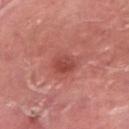Impression:
The lesion was tiled from a total-body skin photograph and was not biopsied.
Background:
About 2.5 mm across. A 15 mm close-up tile from a total-body photography series done for melanoma screening. From the leg. This is a white-light tile. The patient is a male roughly 40 years of age.